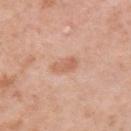Imaged during a routine full-body skin examination; the lesion was not biopsied and no histopathology is available.
The tile uses white-light illumination.
A close-up tile cropped from a whole-body skin photograph, about 15 mm across.
A female subject, roughly 40 years of age.
Located on the right upper arm.